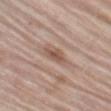biopsy status = imaged on a skin check; not biopsied
size = ~3 mm (longest diameter)
acquisition = total-body-photography crop, ~15 mm field of view
illumination = white-light
anatomic site = the left thigh
patient = male, about 80 years old